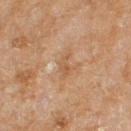Q: Was a biopsy performed?
A: catalogued during a skin exam; not biopsied
Q: What is the imaging modality?
A: ~15 mm crop, total-body skin-cancer survey
Q: What is the anatomic site?
A: the right thigh
Q: Illumination type?
A: cross-polarized illumination
Q: What is the lesion's diameter?
A: ~3 mm (longest diameter)
Q: Who is the patient?
A: female, about 60 years old